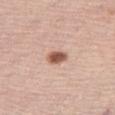Recorded during total-body skin imaging; not selected for excision or biopsy. Measured at roughly 2.5 mm in maximum diameter. The tile uses white-light illumination. Cropped from a whole-body photographic skin survey; the tile spans about 15 mm. A female subject about 65 years old. The lesion is on the right thigh. An algorithmic analysis of the crop reported a lesion area of about 4 mm², a shape eccentricity near 0.7, and a shape-asymmetry score of about 0.2 (0 = symmetric).